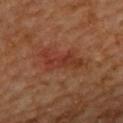workup — no biopsy performed (imaged during a skin exam) | subject — male, in their 60s | imaging modality — 15 mm crop, total-body photography | lighting — cross-polarized | automated lesion analysis — a classifier nevus-likeness of about 0/100 and lesion-presence confidence of about 100/100 | anatomic site — the upper back.